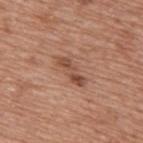Impression: Imaged during a routine full-body skin examination; the lesion was not biopsied and no histopathology is available. Context: A male subject, aged 73 to 77. The lesion is on the upper back. An algorithmic analysis of the crop reported an outline eccentricity of about 0.95 (0 = round, 1 = elongated). And it measured an average lesion color of about L≈48 a*≈22 b*≈29 (CIELAB), a lesion–skin lightness drop of about 10, and a lesion-to-skin contrast of about 7.5 (normalized; higher = more distinct). And it measured border irregularity of about 5.5 on a 0–10 scale, a within-lesion color-variation index near 4.5/10, and radial color variation of about 1.5. Cropped from a whole-body photographic skin survey; the tile spans about 15 mm.